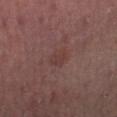biopsy_status: not biopsied; imaged during a skin examination
site: left lower leg
image:
  source: total-body photography crop
  field_of_view_mm: 15
patient:
  sex: male
  age_approx: 55